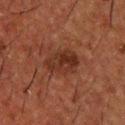Impression:
Captured during whole-body skin photography for melanoma surveillance; the lesion was not biopsied.
Context:
A male patient aged approximately 50. Cropped from a whole-body photographic skin survey; the tile spans about 15 mm. About 4.5 mm across. The lesion is on the upper back.